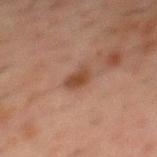Imaged during a routine full-body skin examination; the lesion was not biopsied and no histopathology is available. An algorithmic analysis of the crop reported a shape-asymmetry score of about 0.35 (0 = symmetric). And it measured an average lesion color of about L≈35 a*≈18 b*≈24 (CIELAB), about 8 CIELAB-L* units darker than the surrounding skin, and a normalized lesion–skin contrast near 8. And it measured a border-irregularity index near 3/10, a within-lesion color-variation index near 2/10, and peripheral color asymmetry of about 0.5. It also reported a nevus-likeness score of about 85/100 and lesion-presence confidence of about 100/100. Located on the mid back. Longest diameter approximately 3 mm. Captured under cross-polarized illumination. A male patient approximately 50 years of age. A lesion tile, about 15 mm wide, cut from a 3D total-body photograph.